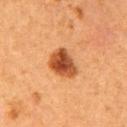Q: Is there a histopathology result?
A: no biopsy performed (imaged during a skin exam)
Q: Lesion location?
A: the right upper arm
Q: What is the lesion's diameter?
A: ~4 mm (longest diameter)
Q: Illumination type?
A: cross-polarized illumination
Q: How was this image acquired?
A: total-body-photography crop, ~15 mm field of view
Q: What are the patient's age and sex?
A: female, about 40 years old